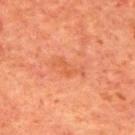Case summary:
* image — ~15 mm crop, total-body skin-cancer survey
* subject — male, aged 63–67
* TBP lesion metrics — a lesion area of about 5 mm², a shape eccentricity near 0.9, and a symmetry-axis asymmetry near 0.55; a border-irregularity rating of about 7/10, internal color variation of about 1 on a 0–10 scale, and a peripheral color-asymmetry measure near 0
* anatomic site — the upper back